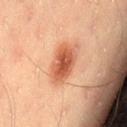biopsy_status: not biopsied; imaged during a skin examination
site: leg
patient:
  sex: male
  age_approx: 45
lighting: cross-polarized
image:
  source: total-body photography crop
  field_of_view_mm: 15